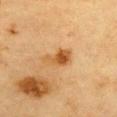Recorded during total-body skin imaging; not selected for excision or biopsy.
From the chest.
Cropped from a whole-body photographic skin survey; the tile spans about 15 mm.
The lesion's longest dimension is about 4 mm.
Captured under cross-polarized illumination.
A female patient, aged 53–57.